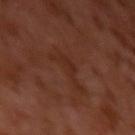From the left upper arm. A male subject, aged 28 to 32. A lesion tile, about 15 mm wide, cut from a 3D total-body photograph. The tile uses cross-polarized illumination. Measured at roughly 4.5 mm in maximum diameter.